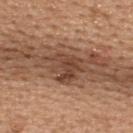Notes:
- notes · no biopsy performed (imaged during a skin exam)
- site · the upper back
- subject · female, approximately 45 years of age
- diameter · ~4.5 mm (longest diameter)
- automated lesion analysis · a shape eccentricity near 0.65 and two-axis asymmetry of about 0.35; a lesion–skin lightness drop of about 11 and a lesion-to-skin contrast of about 8.5 (normalized; higher = more distinct); a border-irregularity rating of about 5.5/10; an automated nevus-likeness rating near 35 out of 100 and a detector confidence of about 100 out of 100 that the crop contains a lesion
- image source · total-body-photography crop, ~15 mm field of view
- tile lighting · white-light illumination